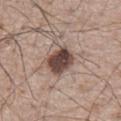Impression:
The lesion was tiled from a total-body skin photograph and was not biopsied.
Acquisition and patient details:
A male patient in their mid-60s. Imaged with white-light lighting. A close-up tile cropped from a whole-body skin photograph, about 15 mm across. Located on the front of the torso. Longest diameter approximately 3.5 mm. The total-body-photography lesion software estimated an area of roughly 10 mm² and two-axis asymmetry of about 0.15. It also reported a mean CIELAB color near L≈45 a*≈17 b*≈22 and roughly 17 lightness units darker than nearby skin. The software also gave a border-irregularity index near 1.5/10 and a within-lesion color-variation index near 5.5/10.